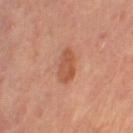biopsy_status: not biopsied; imaged during a skin examination